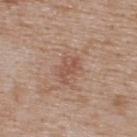follow-up: no biopsy performed (imaged during a skin exam); automated lesion analysis: an area of roughly 5 mm², a shape eccentricity near 0.8, and a symmetry-axis asymmetry near 0.35; body site: the back; image source: ~15 mm crop, total-body skin-cancer survey; patient: male, in their mid- to late 70s; size: ≈3.5 mm; illumination: white-light illumination.